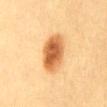{"lesion_size": {"long_diameter_mm_approx": 5.5}, "site": "chest", "patient": {"sex": "female", "age_approx": 20}, "automated_metrics": {"area_mm2_approx": 14.0, "eccentricity": 0.85, "shape_asymmetry": 0.15, "cielab_L": 55, "cielab_a": 23, "cielab_b": 40, "vs_skin_darker_L": 16.0, "vs_skin_contrast_norm": 10.5, "lesion_detection_confidence_0_100": 100}, "image": {"source": "total-body photography crop", "field_of_view_mm": 15}, "lighting": "cross-polarized"}